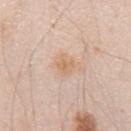| key | value |
|---|---|
| follow-up | imaged on a skin check; not biopsied |
| subject | male, in their mid- to late 40s |
| tile lighting | white-light illumination |
| image | ~15 mm crop, total-body skin-cancer survey |
| lesion size | about 2.5 mm |
| site | the chest |